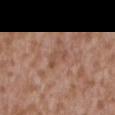Q: Was this lesion biopsied?
A: total-body-photography surveillance lesion; no biopsy
Q: What kind of image is this?
A: 15 mm crop, total-body photography
Q: What are the patient's age and sex?
A: male, roughly 45 years of age
Q: How large is the lesion?
A: ~3 mm (longest diameter)
Q: Where on the body is the lesion?
A: the mid back